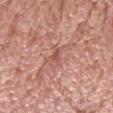Recorded during total-body skin imaging; not selected for excision or biopsy. The recorded lesion diameter is about 2.5 mm. A male patient about 60 years old. On the head or neck. Captured under white-light illumination. A 15 mm close-up extracted from a 3D total-body photography capture.